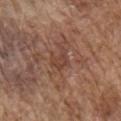This lesion was catalogued during total-body skin photography and was not selected for biopsy. A male subject, roughly 75 years of age. Measured at roughly 2.5 mm in maximum diameter. From the right upper arm. This image is a 15 mm lesion crop taken from a total-body photograph. Automated image analysis of the tile measured a shape eccentricity near 0.7 and two-axis asymmetry of about 0.45. The software also gave border irregularity of about 4.5 on a 0–10 scale and internal color variation of about 1.5 on a 0–10 scale. And it measured a classifier nevus-likeness of about 0/100.